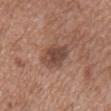Q: Was this lesion biopsied?
A: no biopsy performed (imaged during a skin exam)
Q: What is the anatomic site?
A: the chest
Q: Who is the patient?
A: female, aged 38 to 42
Q: What did automated image analysis measure?
A: a footprint of about 8.5 mm², an eccentricity of roughly 0.8, and a shape-asymmetry score of about 0.2 (0 = symmetric); a border-irregularity index near 2.5/10, a within-lesion color-variation index near 3/10, and radial color variation of about 1
Q: Illumination type?
A: white-light
Q: What is the imaging modality?
A: 15 mm crop, total-body photography